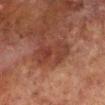| field | value |
|---|---|
| biopsy status | total-body-photography surveillance lesion; no biopsy |
| acquisition | total-body-photography crop, ~15 mm field of view |
| illumination | cross-polarized |
| anatomic site | the right lower leg |
| automated lesion analysis | border irregularity of about 4.5 on a 0–10 scale, a within-lesion color-variation index near 4/10, and radial color variation of about 1.5 |
| patient | male, aged 68 to 72 |